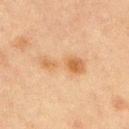location: the chest; image source: total-body-photography crop, ~15 mm field of view; subject: male, aged around 65.